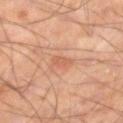A male subject, approximately 55 years of age. A lesion tile, about 15 mm wide, cut from a 3D total-body photograph. The lesion is located on the right lower leg.